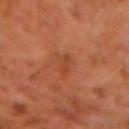No biopsy was performed on this lesion — it was imaged during a full skin examination and was not determined to be concerning.
This image is a 15 mm lesion crop taken from a total-body photograph.
Automated tile analysis of the lesion measured an area of roughly 3.5 mm², an eccentricity of roughly 0.9, and a symmetry-axis asymmetry near 0.35.
The lesion is on the left forearm.
A male subject aged 58 to 62.